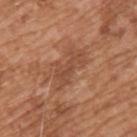workup: total-body-photography surveillance lesion; no biopsy | lighting: white-light | automated metrics: a nevus-likeness score of about 0/100 | lesion size: ≈5.5 mm | patient: male, aged 63 to 67 | image source: total-body-photography crop, ~15 mm field of view | anatomic site: the upper back.